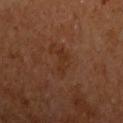Clinical impression: Recorded during total-body skin imaging; not selected for excision or biopsy. Acquisition and patient details: Captured under cross-polarized illumination. A region of skin cropped from a whole-body photographic capture, roughly 15 mm wide. A male subject aged 63–67. Approximately 3.5 mm at its widest. On the left upper arm.